The patient is a male roughly 70 years of age; measured at roughly 5.5 mm in maximum diameter; located on the front of the torso; this is a white-light tile; a roughly 15 mm field-of-view crop from a total-body skin photograph; The lesion-visualizer software estimated an eccentricity of roughly 0.65 and a symmetry-axis asymmetry near 0.25. The analysis additionally found an automated nevus-likeness rating near 5 out of 100 and a lesion-detection confidence of about 100/100: 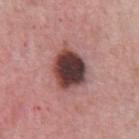Notes:
– diagnosis — a dysplastic (Clark) nevus